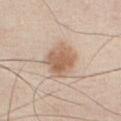Clinical impression:
The lesion was tiled from a total-body skin photograph and was not biopsied.
Background:
From the chest. An algorithmic analysis of the crop reported a footprint of about 14 mm², a shape eccentricity near 0.65, and a symmetry-axis asymmetry near 0.15. And it measured about 12 CIELAB-L* units darker than the surrounding skin and a lesion-to-skin contrast of about 8 (normalized; higher = more distinct). The analysis additionally found a border-irregularity index near 1.5/10, a within-lesion color-variation index near 4/10, and radial color variation of about 1. A region of skin cropped from a whole-body photographic capture, roughly 15 mm wide. The patient is a male aged around 45.